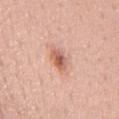notes: imaged on a skin check; not biopsied | body site: the back | illumination: white-light illumination | automated lesion analysis: an area of roughly 5 mm², an outline eccentricity of about 0.8 (0 = round, 1 = elongated), and a symmetry-axis asymmetry near 0.15; a lesion color around L≈61 a*≈25 b*≈30 in CIELAB and a normalized lesion–skin contrast near 8 | image source: ~15 mm crop, total-body skin-cancer survey | subject: female, roughly 45 years of age.